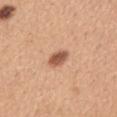This lesion was catalogued during total-body skin photography and was not selected for biopsy. The patient is a female aged approximately 30. Imaged with white-light lighting. Cropped from a total-body skin-imaging series; the visible field is about 15 mm. The lesion is on the mid back.